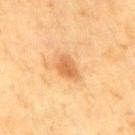Notes:
• follow-up · no biopsy performed (imaged during a skin exam)
• image source · ~15 mm tile from a whole-body skin photo
• anatomic site · the left upper arm
• tile lighting · cross-polarized illumination
• patient · male, about 70 years old